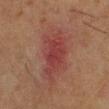Q: Is there a histopathology result?
A: imaged on a skin check; not biopsied
Q: Illumination type?
A: cross-polarized
Q: What is the lesion's diameter?
A: ≈6.5 mm
Q: What is the imaging modality?
A: ~15 mm crop, total-body skin-cancer survey
Q: Lesion location?
A: the right lower leg
Q: Who is the patient?
A: female, aged 48 to 52